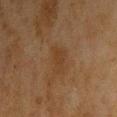Q: Is there a histopathology result?
A: catalogued during a skin exam; not biopsied
Q: Lesion location?
A: the front of the torso
Q: What is the imaging modality?
A: ~15 mm crop, total-body skin-cancer survey
Q: Lesion size?
A: ~3.5 mm (longest diameter)
Q: What lighting was used for the tile?
A: cross-polarized illumination
Q: Patient demographics?
A: male, in their mid-40s